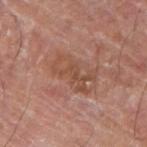Case summary:
• notes · total-body-photography surveillance lesion; no biopsy
• subject · male, in their 60s
• image · ~15 mm tile from a whole-body skin photo
• location · the right upper arm
• diameter · about 5 mm